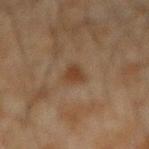No biopsy was performed on this lesion — it was imaged during a full skin examination and was not determined to be concerning. Cropped from a total-body skin-imaging series; the visible field is about 15 mm. From the left forearm. The recorded lesion diameter is about 2.5 mm. The total-body-photography lesion software estimated a border-irregularity index near 3/10, a within-lesion color-variation index near 1.5/10, and a peripheral color-asymmetry measure near 0.5. And it measured an automated nevus-likeness rating near 75 out of 100. Imaged with cross-polarized lighting. The subject is a male aged around 45.